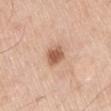No biopsy was performed on this lesion — it was imaged during a full skin examination and was not determined to be concerning.
Measured at roughly 2.5 mm in maximum diameter.
This image is a 15 mm lesion crop taken from a total-body photograph.
The lesion-visualizer software estimated a mean CIELAB color near L≈58 a*≈22 b*≈32, roughly 14 lightness units darker than nearby skin, and a normalized lesion–skin contrast near 8.5. The analysis additionally found a classifier nevus-likeness of about 95/100.
Imaged with white-light lighting.
On the right thigh.
A male subject about 60 years old.